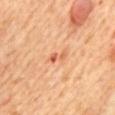biopsy_status: not biopsied; imaged during a skin examination
patient:
  sex: female
  age_approx: 65
automated_metrics:
  area_mm2_approx: 2.5
  shape_asymmetry: 0.35
  border_irregularity_0_10: 4.5
  color_variation_0_10: 0.0
  peripheral_color_asymmetry: 0.0
  lesion_detection_confidence_0_100: 100
image:
  source: total-body photography crop
  field_of_view_mm: 15
lesion_size:
  long_diameter_mm_approx: 2.5
site: back
lighting: cross-polarized